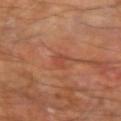workup=imaged on a skin check; not biopsied | image=15 mm crop, total-body photography | anatomic site=the arm | subject=male, approximately 70 years of age | lesion size=about 2.5 mm.